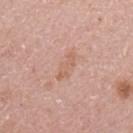<case>
  <biopsy_status>not biopsied; imaged during a skin examination</biopsy_status>
  <image>
    <source>total-body photography crop</source>
    <field_of_view_mm>15</field_of_view_mm>
  </image>
  <lighting>white-light</lighting>
  <automated_metrics>
    <border_irregularity_0_10>4.0</border_irregularity_0_10>
    <color_variation_0_10>1.5</color_variation_0_10>
    <peripheral_color_asymmetry>0.0</peripheral_color_asymmetry>
  </automated_metrics>
  <site>left upper arm</site>
  <patient>
    <sex>female</sex>
    <age_approx>50</age_approx>
  </patient>
  <lesion_size>
    <long_diameter_mm_approx>4.0</long_diameter_mm_approx>
  </lesion_size>
</case>